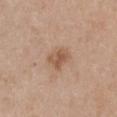workup=total-body-photography surveillance lesion; no biopsy
illumination=white-light illumination
location=the chest
image source=15 mm crop, total-body photography
patient=female, aged around 40